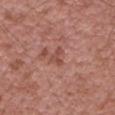notes: catalogued during a skin exam; not biopsied
acquisition: ~15 mm crop, total-body skin-cancer survey
lesion size: about 2.5 mm
image-analysis metrics: a lesion color around L≈49 a*≈25 b*≈27 in CIELAB, a lesion–skin lightness drop of about 8, and a lesion-to-skin contrast of about 6 (normalized; higher = more distinct); border irregularity of about 6.5 on a 0–10 scale, a color-variation rating of about 0/10, and a peripheral color-asymmetry measure near 0
subject: male, aged 48–52
site: the left upper arm
illumination: white-light illumination